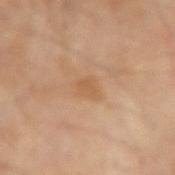The lesion was tiled from a total-body skin photograph and was not biopsied. This image is a 15 mm lesion crop taken from a total-body photograph. The tile uses cross-polarized illumination. The lesion is located on the right forearm. Automated image analysis of the tile measured a lesion area of about 4 mm², an eccentricity of roughly 0.65, and a symmetry-axis asymmetry near 0.3. It also reported a border-irregularity rating of about 3/10, a color-variation rating of about 1/10, and peripheral color asymmetry of about 0.5. A male subject about 70 years old.